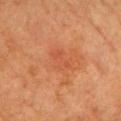Clinical impression: The lesion was tiled from a total-body skin photograph and was not biopsied. Background: About 3 mm across. Imaged with cross-polarized lighting. A female subject, in their mid-60s. Automated image analysis of the tile measured a normalized lesion–skin contrast near 3.5. The software also gave border irregularity of about 4.5 on a 0–10 scale, internal color variation of about 2.5 on a 0–10 scale, and radial color variation of about 0.5. The analysis additionally found a classifier nevus-likeness of about 15/100 and lesion-presence confidence of about 100/100. The lesion is on the head or neck. A roughly 15 mm field-of-view crop from a total-body skin photograph.